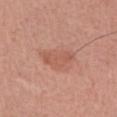Impression:
No biopsy was performed on this lesion — it was imaged during a full skin examination and was not determined to be concerning.
Clinical summary:
The tile uses white-light illumination. The recorded lesion diameter is about 4 mm. A region of skin cropped from a whole-body photographic capture, roughly 15 mm wide. The patient is a female approximately 50 years of age. Located on the left forearm.